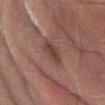Image and clinical context:
Captured under white-light illumination. The lesion is located on the left forearm. The recorded lesion diameter is about 3.5 mm. A male subject approximately 70 years of age. This image is a 15 mm lesion crop taken from a total-body photograph. An algorithmic analysis of the crop reported a classifier nevus-likeness of about 0/100 and a lesion-detection confidence of about 95/100.